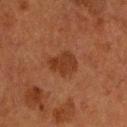Part of a total-body skin-imaging series; this lesion was reviewed on a skin check and was not flagged for biopsy.
A close-up tile cropped from a whole-body skin photograph, about 15 mm across.
The lesion is on the head or neck.
This is a cross-polarized tile.
A male patient, aged around 60.
The recorded lesion diameter is about 4 mm.